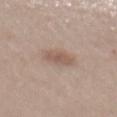  biopsy_status: not biopsied; imaged during a skin examination
  site: abdomen
  lesion_size:
    long_diameter_mm_approx: 3.0
  patient:
    sex: female
    age_approx: 55
  automated_metrics:
    area_mm2_approx: 5.0
    eccentricity: 0.65
    shape_asymmetry: 0.15
    border_irregularity_0_10: 1.5
    color_variation_0_10: 2.5
  lighting: white-light
  image:
    source: total-body photography crop
    field_of_view_mm: 15